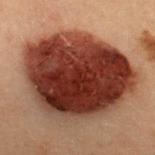notes = imaged on a skin check; not biopsied | lesion diameter = ≈11 mm | site = the upper back | subject = female, approximately 30 years of age | acquisition = ~15 mm tile from a whole-body skin photo | illumination = cross-polarized.